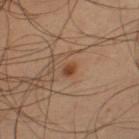The lesion was tiled from a total-body skin photograph and was not biopsied.
A region of skin cropped from a whole-body photographic capture, roughly 15 mm wide.
This is a cross-polarized tile.
From the left thigh.
A male patient, about 40 years old.
About 1.5 mm across.